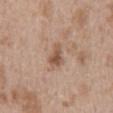patient: male, aged 48–52
size: about 3 mm
tile lighting: white-light illumination
imaging modality: ~15 mm tile from a whole-body skin photo
body site: the back